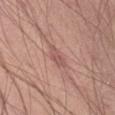workup: catalogued during a skin exam; not biopsied | acquisition: ~15 mm tile from a whole-body skin photo | location: the abdomen | subject: male, in their mid-50s.